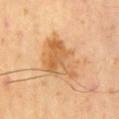Notes:
- workup: catalogued during a skin exam; not biopsied
- image-analysis metrics: an outline eccentricity of about 0.65 (0 = round, 1 = elongated) and a symmetry-axis asymmetry near 0.3; a lesion color around L≈63 a*≈21 b*≈42 in CIELAB and a lesion–skin lightness drop of about 10; border irregularity of about 4 on a 0–10 scale, a color-variation rating of about 6.5/10, and peripheral color asymmetry of about 2; a nevus-likeness score of about 30/100 and lesion-presence confidence of about 100/100
- image: total-body-photography crop, ~15 mm field of view
- lesion diameter: ≈6 mm
- site: the back
- patient: male, aged approximately 65